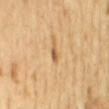notes=no biopsy performed (imaged during a skin exam)
subject=female, in their mid-50s
body site=the mid back
diameter=~2 mm (longest diameter)
illumination=cross-polarized illumination
acquisition=total-body-photography crop, ~15 mm field of view